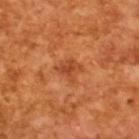<lesion>
<biopsy_status>not biopsied; imaged during a skin examination</biopsy_status>
<lesion_size>
  <long_diameter_mm_approx>2.5</long_diameter_mm_approx>
</lesion_size>
<lighting>cross-polarized</lighting>
<patient>
  <sex>male</sex>
  <age_approx>65</age_approx>
</patient>
<image>
  <source>total-body photography crop</source>
  <field_of_view_mm>15</field_of_view_mm>
</image>
</lesion>